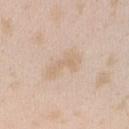Impression:
The lesion was photographed on a routine skin check and not biopsied; there is no pathology result.
Image and clinical context:
Captured under white-light illumination. The lesion-visualizer software estimated an average lesion color of about L≈69 a*≈14 b*≈31 (CIELAB) and roughly 6 lightness units darker than nearby skin. From the right forearm. Measured at roughly 4.5 mm in maximum diameter. A female patient, roughly 25 years of age. A region of skin cropped from a whole-body photographic capture, roughly 15 mm wide.